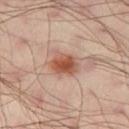Recorded during total-body skin imaging; not selected for excision or biopsy.
This image is a 15 mm lesion crop taken from a total-body photograph.
A male patient in their 40s.
Located on the right thigh.